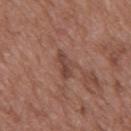Q: Was a biopsy performed?
A: total-body-photography surveillance lesion; no biopsy
Q: What are the patient's age and sex?
A: male, aged 48–52
Q: What is the anatomic site?
A: the mid back
Q: How was this image acquired?
A: ~15 mm crop, total-body skin-cancer survey